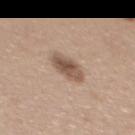Case summary:
• notes: no biopsy performed (imaged during a skin exam)
• image: ~15 mm crop, total-body skin-cancer survey
• patient: female, aged approximately 40
• site: the upper back